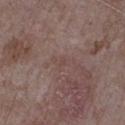workup=no biopsy performed (imaged during a skin exam) | lighting=white-light | TBP lesion metrics=a mean CIELAB color near L≈44 a*≈17 b*≈20, a lesion–skin lightness drop of about 4, and a normalized lesion–skin contrast near 3.5; a nevus-likeness score of about 0/100 and a detector confidence of about 95 out of 100 that the crop contains a lesion | image=total-body-photography crop, ~15 mm field of view | patient=male, about 65 years old | lesion diameter=≈1.5 mm | body site=the left upper arm.